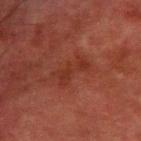Acquisition and patient details: Cropped from a whole-body photographic skin survey; the tile spans about 15 mm. A male patient, aged approximately 80. The lesion is on the left forearm.